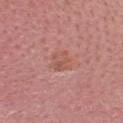* workup · catalogued during a skin exam; not biopsied
* imaging modality · total-body-photography crop, ~15 mm field of view
* tile lighting · white-light illumination
* patient · female, aged 48 to 52
* lesion size · ≈3 mm
* location · the head or neck